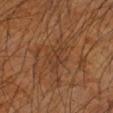Q: Is there a histopathology result?
A: catalogued during a skin exam; not biopsied
Q: Illumination type?
A: cross-polarized illumination
Q: Lesion location?
A: the left forearm
Q: Lesion size?
A: ~4 mm (longest diameter)
Q: Automated lesion metrics?
A: a border-irregularity rating of about 5.5/10, a color-variation rating of about 2/10, and peripheral color asymmetry of about 0.5; a nevus-likeness score of about 0/100 and a lesion-detection confidence of about 60/100
Q: What kind of image is this?
A: 15 mm crop, total-body photography
Q: What are the patient's age and sex?
A: male, aged 58 to 62